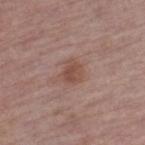<case>
  <lighting>white-light</lighting>
  <patient>
    <sex>male</sex>
    <age_approx>75</age_approx>
  </patient>
  <automated_metrics>
    <border_irregularity_0_10>2.0</border_irregularity_0_10>
    <color_variation_0_10>3.0</color_variation_0_10>
    <nevus_likeness_0_100>40</nevus_likeness_0_100>
    <lesion_detection_confidence_0_100>100</lesion_detection_confidence_0_100>
  </automated_metrics>
  <image>
    <source>total-body photography crop</source>
    <field_of_view_mm>15</field_of_view_mm>
  </image>
  <lesion_size>
    <long_diameter_mm_approx>2.5</long_diameter_mm_approx>
  </lesion_size>
  <site>left thigh</site>
</case>